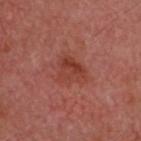workup — no biopsy performed (imaged during a skin exam) | image-analysis metrics — a mean CIELAB color near L≈41 a*≈29 b*≈29 and a normalized lesion–skin contrast near 6.5; lesion-presence confidence of about 100/100 | image — ~15 mm tile from a whole-body skin photo | lesion size — about 3.5 mm | subject — male, in their mid-60s | body site — the head or neck | lighting — cross-polarized illumination.